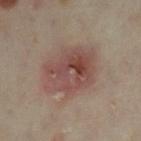Assessment: No biopsy was performed on this lesion — it was imaged during a full skin examination and was not determined to be concerning. Background: Located on the left lower leg. This image is a 15 mm lesion crop taken from a total-body photograph. Approximately 7 mm at its widest. The lesion-visualizer software estimated an area of roughly 24 mm², an eccentricity of roughly 0.7, and a symmetry-axis asymmetry near 0.15. A female subject, roughly 35 years of age. The tile uses cross-polarized illumination.